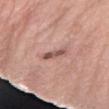The lesion was photographed on a routine skin check and not biopsied; there is no pathology result. Captured under white-light illumination. The subject is a female aged around 60. Located on the left forearm. The recorded lesion diameter is about 3.5 mm. Cropped from a total-body skin-imaging series; the visible field is about 15 mm.